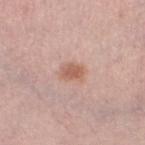{
  "biopsy_status": "not biopsied; imaged during a skin examination",
  "patient": {
    "sex": "female",
    "age_approx": 65
  },
  "lighting": "white-light",
  "lesion_size": {
    "long_diameter_mm_approx": 3.0
  },
  "image": {
    "source": "total-body photography crop",
    "field_of_view_mm": 15
  },
  "site": "left thigh"
}